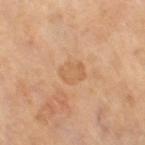| field | value |
|---|---|
| follow-up | imaged on a skin check; not biopsied |
| patient | female, roughly 65 years of age |
| TBP lesion metrics | an area of roughly 6 mm², a shape eccentricity near 0.5, and a symmetry-axis asymmetry near 0.25; an average lesion color of about L≈59 a*≈20 b*≈36 (CIELAB) and a lesion–skin lightness drop of about 6; a nevus-likeness score of about 0/100 |
| lighting | cross-polarized |
| size | ~3 mm (longest diameter) |
| body site | the right thigh |
| acquisition | 15 mm crop, total-body photography |